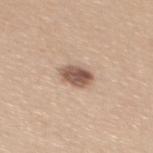workup: catalogued during a skin exam; not biopsied
location: the upper back
patient: female, approximately 45 years of age
imaging modality: ~15 mm tile from a whole-body skin photo
automated lesion analysis: a lesion area of about 6.5 mm², an outline eccentricity of about 0.75 (0 = round, 1 = elongated), and two-axis asymmetry of about 0.15; a lesion color around L≈55 a*≈18 b*≈27 in CIELAB, roughly 16 lightness units darker than nearby skin, and a normalized lesion–skin contrast near 10; a lesion-detection confidence of about 100/100
size: ~3.5 mm (longest diameter)